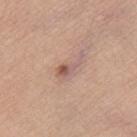Image and clinical context:
The tile uses white-light illumination. Located on the leg. A female patient aged 58 to 62. A 15 mm crop from a total-body photograph taken for skin-cancer surveillance.